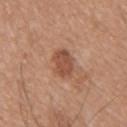Assessment: Part of a total-body skin-imaging series; this lesion was reviewed on a skin check and was not flagged for biopsy. Acquisition and patient details: The total-body-photography lesion software estimated an automated nevus-likeness rating near 30 out of 100 and a lesion-detection confidence of about 100/100. The lesion's longest dimension is about 3.5 mm. Located on the upper back. A roughly 15 mm field-of-view crop from a total-body skin photograph. The patient is a male aged 38–42.